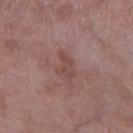Imaged during a routine full-body skin examination; the lesion was not biopsied and no histopathology is available. On the right lower leg. The total-body-photography lesion software estimated internal color variation of about 1.5 on a 0–10 scale. The recorded lesion diameter is about 3.5 mm. The tile uses white-light illumination. A female subject, aged 68 to 72. Cropped from a total-body skin-imaging series; the visible field is about 15 mm.